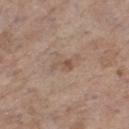Recorded during total-body skin imaging; not selected for excision or biopsy.
Approximately 2.5 mm at its widest.
Located on the right lower leg.
A 15 mm close-up extracted from a 3D total-body photography capture.
The patient is a female about 85 years old or older.